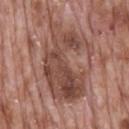<lesion>
<biopsy_status>not biopsied; imaged during a skin examination</biopsy_status>
<patient>
  <sex>male</sex>
  <age_approx>70</age_approx>
</patient>
<image>
  <source>total-body photography crop</source>
  <field_of_view_mm>15</field_of_view_mm>
</image>
<site>mid back</site>
<lighting>white-light</lighting>
<lesion_size>
  <long_diameter_mm_approx>8.5</long_diameter_mm_approx>
</lesion_size>
</lesion>